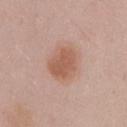<record>
  <site>chest</site>
  <automated_metrics>
    <area_mm2_approx>11.0</area_mm2_approx>
    <eccentricity>0.65</eccentricity>
    <shape_asymmetry>0.15</shape_asymmetry>
    <border_irregularity_0_10>2.0</border_irregularity_0_10>
    <color_variation_0_10>2.5</color_variation_0_10>
    <peripheral_color_asymmetry>1.0</peripheral_color_asymmetry>
    <nevus_likeness_0_100>90</nevus_likeness_0_100>
    <lesion_detection_confidence_0_100>100</lesion_detection_confidence_0_100>
  </automated_metrics>
  <lesion_size>
    <long_diameter_mm_approx>4.5</long_diameter_mm_approx>
  </lesion_size>
  <image>
    <source>total-body photography crop</source>
    <field_of_view_mm>15</field_of_view_mm>
  </image>
  <patient>
    <sex>male</sex>
    <age_approx>55</age_approx>
  </patient>
</record>